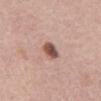biopsy status = catalogued during a skin exam; not biopsied | lesion diameter = ~2.5 mm (longest diameter) | subject = female, in their 60s | lighting = white-light illumination | image source = 15 mm crop, total-body photography | automated lesion analysis = a lesion color around L≈51 a*≈21 b*≈25 in CIELAB and about 16 CIELAB-L* units darker than the surrounding skin; border irregularity of about 2.5 on a 0–10 scale | site = the front of the torso.